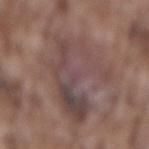Imaged during a routine full-body skin examination; the lesion was not biopsied and no histopathology is available.
Automated image analysis of the tile measured a lesion area of about 36 mm², an eccentricity of roughly 0.75, and two-axis asymmetry of about 0.55. The analysis additionally found a color-variation rating of about 7/10 and peripheral color asymmetry of about 2.
A region of skin cropped from a whole-body photographic capture, roughly 15 mm wide.
A male subject aged 73 to 77.
From the lower back.
The tile uses white-light illumination.
The recorded lesion diameter is about 8.5 mm.